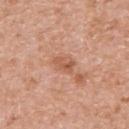Notes:
• automated lesion analysis: a nevus-likeness score of about 0/100 and a lesion-detection confidence of about 100/100
• imaging modality: ~15 mm crop, total-body skin-cancer survey
• patient: female, aged approximately 70
• body site: the right upper arm
• lighting: white-light
• size: ~2.5 mm (longest diameter)